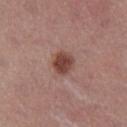Clinical impression:
This lesion was catalogued during total-body skin photography and was not selected for biopsy.
Context:
A region of skin cropped from a whole-body photographic capture, roughly 15 mm wide. The lesion-visualizer software estimated a shape eccentricity near 0.5 and a shape-asymmetry score of about 0.2 (0 = symmetric). The software also gave a mean CIELAB color near L≈43 a*≈23 b*≈24, a lesion–skin lightness drop of about 13, and a normalized lesion–skin contrast near 10. The software also gave a within-lesion color-variation index near 2.5/10 and radial color variation of about 1. This is a white-light tile. From the leg. The patient is a female approximately 55 years of age. Longest diameter approximately 3 mm.